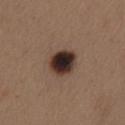Impression:
Captured during whole-body skin photography for melanoma surveillance; the lesion was not biopsied.